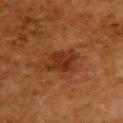Imaged during a routine full-body skin examination; the lesion was not biopsied and no histopathology is available. A 15 mm crop from a total-body photograph taken for skin-cancer surveillance. The lesion is on the back. A female subject aged around 50.